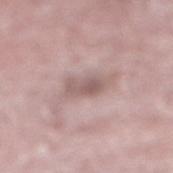Clinical impression:
This lesion was catalogued during total-body skin photography and was not selected for biopsy.
Clinical summary:
The subject is a male in their mid-70s. A lesion tile, about 15 mm wide, cut from a 3D total-body photograph. Longest diameter approximately 4 mm. Located on the right lower leg. The tile uses white-light illumination.